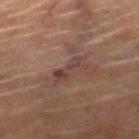The lesion was tiled from a total-body skin photograph and was not biopsied.
A lesion tile, about 15 mm wide, cut from a 3D total-body photograph.
The recorded lesion diameter is about 3.5 mm.
The lesion is on the right lower leg.
This is a cross-polarized tile.
The lesion-visualizer software estimated roughly 7 lightness units darker than nearby skin and a normalized lesion–skin contrast near 7. The analysis additionally found internal color variation of about 1 on a 0–10 scale and a peripheral color-asymmetry measure near 0. It also reported a classifier nevus-likeness of about 0/100.
The patient is a male aged approximately 85 or older.